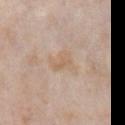Findings:
- biopsy status · total-body-photography surveillance lesion; no biopsy
- image · total-body-photography crop, ~15 mm field of view
- patient · female, aged 63–67
- illumination · white-light illumination
- size · ≈3 mm
- site · the chest
- automated lesion analysis · a lesion color around L≈63 a*≈15 b*≈31 in CIELAB, a lesion–skin lightness drop of about 5, and a normalized lesion–skin contrast near 5.5